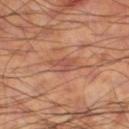Q: Is there a histopathology result?
A: imaged on a skin check; not biopsied
Q: Where on the body is the lesion?
A: the left thigh
Q: Patient demographics?
A: male, aged 58 to 62
Q: What kind of image is this?
A: total-body-photography crop, ~15 mm field of view
Q: How was the tile lit?
A: cross-polarized
Q: How large is the lesion?
A: about 2.5 mm